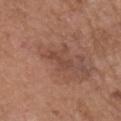Captured during whole-body skin photography for melanoma surveillance; the lesion was not biopsied. The lesion is on the head or neck. A male subject aged approximately 55. This is a white-light tile. A close-up tile cropped from a whole-body skin photograph, about 15 mm across. Approximately 3 mm at its widest.